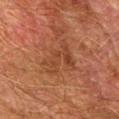Q: Was a biopsy performed?
A: catalogued during a skin exam; not biopsied
Q: What are the patient's age and sex?
A: male, approximately 75 years of age
Q: How was this image acquired?
A: ~15 mm tile from a whole-body skin photo
Q: What is the anatomic site?
A: the right forearm